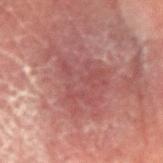Clinical impression:
Recorded during total-body skin imaging; not selected for excision or biopsy.